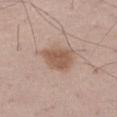biopsy_status: not biopsied; imaged during a skin examination
lesion_size:
  long_diameter_mm_approx: 4.0
site: left thigh
lighting: white-light
automated_metrics:
  cielab_L: 55
  cielab_a: 18
  cielab_b: 28
  vs_skin_darker_L: 11.0
  vs_skin_contrast_norm: 8.0
  border_irregularity_0_10: 2.5
  color_variation_0_10: 2.0
  peripheral_color_asymmetry: 0.5
image:
  source: total-body photography crop
  field_of_view_mm: 15
patient:
  sex: male
  age_approx: 50